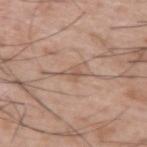Clinical impression: Part of a total-body skin-imaging series; this lesion was reviewed on a skin check and was not flagged for biopsy.